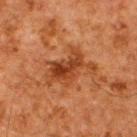Clinical impression:
The lesion was tiled from a total-body skin photograph and was not biopsied.
Acquisition and patient details:
The lesion's longest dimension is about 6 mm. The patient is a male approximately 60 years of age. Cropped from a whole-body photographic skin survey; the tile spans about 15 mm. On the upper back. Automated image analysis of the tile measured an average lesion color of about L≈34 a*≈23 b*≈31 (CIELAB), a lesion–skin lightness drop of about 9, and a normalized border contrast of about 8. The analysis additionally found a nevus-likeness score of about 5/100 and a detector confidence of about 100 out of 100 that the crop contains a lesion. Captured under cross-polarized illumination.